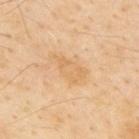Part of a total-body skin-imaging series; this lesion was reviewed on a skin check and was not flagged for biopsy. A roughly 15 mm field-of-view crop from a total-body skin photograph. The subject is a male in their mid-50s. Imaged with cross-polarized lighting. Located on the upper back. About 4.5 mm across. The lesion-visualizer software estimated an average lesion color of about L≈69 a*≈18 b*≈41 (CIELAB). The analysis additionally found border irregularity of about 4 on a 0–10 scale, a within-lesion color-variation index near 2.5/10, and radial color variation of about 0.5. And it measured a lesion-detection confidence of about 100/100.